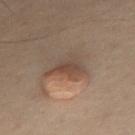* illumination: cross-polarized
* diameter: ≈4 mm
* anatomic site: the mid back
* automated metrics: a border-irregularity index near 5.5/10, internal color variation of about 3.5 on a 0–10 scale, and a peripheral color-asymmetry measure near 1; a classifier nevus-likeness of about 35/100 and a lesion-detection confidence of about 55/100
* acquisition: total-body-photography crop, ~15 mm field of view
* patient: male, about 50 years old